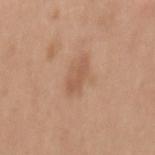Assessment:
No biopsy was performed on this lesion — it was imaged during a full skin examination and was not determined to be concerning.
Image and clinical context:
Longest diameter approximately 3.5 mm. A male patient approximately 30 years of age. Cropped from a total-body skin-imaging series; the visible field is about 15 mm. On the mid back. Imaged with white-light lighting.